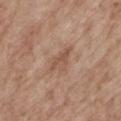No biopsy was performed on this lesion — it was imaged during a full skin examination and was not determined to be concerning.
Measured at roughly 4 mm in maximum diameter.
Automated tile analysis of the lesion measured a lesion area of about 6 mm², an outline eccentricity of about 0.8 (0 = round, 1 = elongated), and a symmetry-axis asymmetry near 0.45. The software also gave a mean CIELAB color near L≈53 a*≈19 b*≈28, a lesion–skin lightness drop of about 8, and a normalized lesion–skin contrast near 5.5. It also reported a border-irregularity index near 4.5/10, internal color variation of about 3 on a 0–10 scale, and radial color variation of about 1.
On the mid back.
A male subject, aged approximately 70.
Cropped from a total-body skin-imaging series; the visible field is about 15 mm.
Captured under white-light illumination.